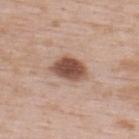notes: total-body-photography surveillance lesion; no biopsy | acquisition: ~15 mm tile from a whole-body skin photo | site: the upper back | patient: male, roughly 50 years of age | size: about 4.5 mm | lighting: white-light.